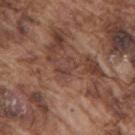{"biopsy_status": "not biopsied; imaged during a skin examination", "patient": {"sex": "male", "age_approx": 75}, "image": {"source": "total-body photography crop", "field_of_view_mm": 15}, "site": "upper back", "lighting": "white-light", "lesion_size": {"long_diameter_mm_approx": 2.5}, "automated_metrics": {"area_mm2_approx": 3.0, "eccentricity": 0.85, "shape_asymmetry": 0.55, "vs_skin_darker_L": 6.0, "nevus_likeness_0_100": 0}}